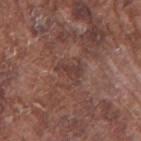Measured at roughly 3 mm in maximum diameter.
A male patient aged approximately 75.
From the right upper arm.
A lesion tile, about 15 mm wide, cut from a 3D total-body photograph.
This is a white-light tile.
Automated image analysis of the tile measured a footprint of about 3.5 mm², an outline eccentricity of about 0.85 (0 = round, 1 = elongated), and a symmetry-axis asymmetry near 0.45. And it measured a lesion color around L≈39 a*≈20 b*≈22 in CIELAB, about 7 CIELAB-L* units darker than the surrounding skin, and a lesion-to-skin contrast of about 6 (normalized; higher = more distinct).